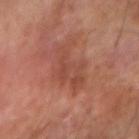{"biopsy_status": "not biopsied; imaged during a skin examination", "lighting": "cross-polarized", "image": {"source": "total-body photography crop", "field_of_view_mm": 15}, "patient": {"sex": "male", "age_approx": 60}, "automated_metrics": {"area_mm2_approx": 13.0, "shape_asymmetry": 0.4, "border_irregularity_0_10": 6.0, "peripheral_color_asymmetry": 1.0, "nevus_likeness_0_100": 0, "lesion_detection_confidence_0_100": 100}, "lesion_size": {"long_diameter_mm_approx": 6.0}, "site": "right forearm"}